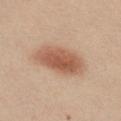The patient is a female aged approximately 40. An algorithmic analysis of the crop reported a footprint of about 15 mm² and a symmetry-axis asymmetry near 0.1. And it measured a lesion color around L≈57 a*≈22 b*≈32 in CIELAB and a lesion–skin lightness drop of about 13. The lesion is located on the chest. A 15 mm close-up extracted from a 3D total-body photography capture.